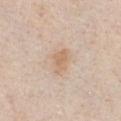No biopsy was performed on this lesion — it was imaged during a full skin examination and was not determined to be concerning.
A male patient roughly 35 years of age.
From the chest.
The tile uses white-light illumination.
Automated image analysis of the tile measured a mean CIELAB color near L≈65 a*≈17 b*≈32 and roughly 8 lightness units darker than nearby skin. It also reported an automated nevus-likeness rating near 40 out of 100 and a detector confidence of about 100 out of 100 that the crop contains a lesion.
A roughly 15 mm field-of-view crop from a total-body skin photograph.